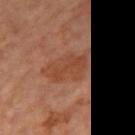Case summary:
• notes · catalogued during a skin exam; not biopsied
• image-analysis metrics · a footprint of about 14 mm² and an outline eccentricity of about 0.8 (0 = round, 1 = elongated)
• subject · female, approximately 65 years of age
• image source · ~15 mm crop, total-body skin-cancer survey
• illumination · cross-polarized illumination
• site · the right thigh
• lesion diameter · ~6 mm (longest diameter)